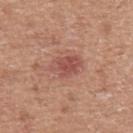Assessment:
The lesion was photographed on a routine skin check and not biopsied; there is no pathology result.
Background:
From the upper back. This is a white-light tile. About 4 mm across. A male patient, in their mid- to late 50s. A roughly 15 mm field-of-view crop from a total-body skin photograph. Automated image analysis of the tile measured an area of roughly 7.5 mm², a shape eccentricity near 0.8, and a shape-asymmetry score of about 0.3 (0 = symmetric). It also reported a border-irregularity rating of about 3.5/10 and internal color variation of about 3 on a 0–10 scale. The software also gave an automated nevus-likeness rating near 0 out of 100 and a detector confidence of about 100 out of 100 that the crop contains a lesion.